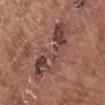• biopsy status — no biopsy performed (imaged during a skin exam)
• subject — male, aged 68–72
• body site — the left upper arm
• imaging modality — total-body-photography crop, ~15 mm field of view
• TBP lesion metrics — a mean CIELAB color near L≈44 a*≈21 b*≈21, a lesion–skin lightness drop of about 9, and a lesion-to-skin contrast of about 8 (normalized; higher = more distinct); an automated nevus-likeness rating near 0 out of 100 and a detector confidence of about 100 out of 100 that the crop contains a lesion
• size — ≈8.5 mm